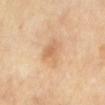{
  "biopsy_status": "not biopsied; imaged during a skin examination",
  "site": "mid back",
  "lighting": "cross-polarized",
  "automated_metrics": {
    "area_mm2_approx": 8.5,
    "shape_asymmetry": 0.25,
    "border_irregularity_0_10": 2.5,
    "color_variation_0_10": 4.0,
    "peripheral_color_asymmetry": 1.0,
    "nevus_likeness_0_100": 5,
    "lesion_detection_confidence_0_100": 100
  },
  "image": {
    "source": "total-body photography crop",
    "field_of_view_mm": 15
  }
}